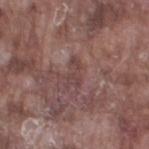Part of a total-body skin-imaging series; this lesion was reviewed on a skin check and was not flagged for biopsy. A 15 mm crop from a total-body photograph taken for skin-cancer surveillance. A male patient aged 73 to 77. The lesion is on the leg.